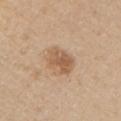* notes · total-body-photography surveillance lesion; no biopsy
* patient · male, in their 40s
* acquisition · ~15 mm tile from a whole-body skin photo
* location · the chest